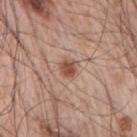Part of a total-body skin-imaging series; this lesion was reviewed on a skin check and was not flagged for biopsy.